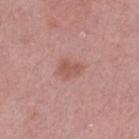- notes — total-body-photography surveillance lesion; no biopsy
- imaging modality — ~15 mm crop, total-body skin-cancer survey
- location — the left thigh
- TBP lesion metrics — a shape eccentricity near 0.7 and a symmetry-axis asymmetry near 0.25; a nevus-likeness score of about 5/100 and a lesion-detection confidence of about 100/100
- subject — female, aged 68–72